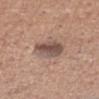<case>
<biopsy_status>not biopsied; imaged during a skin examination</biopsy_status>
<patient>
  <sex>male</sex>
  <age_approx>50</age_approx>
</patient>
<site>right upper arm</site>
<lighting>white-light</lighting>
<image>
  <source>total-body photography crop</source>
  <field_of_view_mm>15</field_of_view_mm>
</image>
<automated_metrics>
  <area_mm2_approx>10.0</area_mm2_approx>
  <eccentricity>0.65</eccentricity>
  <shape_asymmetry>0.15</shape_asymmetry>
  <cielab_L>51</cielab_L>
  <cielab_a>17</cielab_a>
  <cielab_b>23</cielab_b>
  <vs_skin_darker_L>12.0</vs_skin_darker_L>
  <nevus_likeness_0_100>55</nevus_likeness_0_100>
</automated_metrics>
</case>